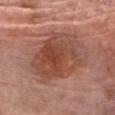• notes · no biopsy performed (imaged during a skin exam)
• patient · male, about 80 years old
• tile lighting · white-light illumination
• lesion diameter · ≈7.5 mm
• site · the chest
• image-analysis metrics · a border-irregularity index near 2.5/10 and a peripheral color-asymmetry measure near 2
• imaging modality · ~15 mm tile from a whole-body skin photo